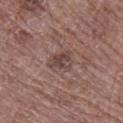Assessment: No biopsy was performed on this lesion — it was imaged during a full skin examination and was not determined to be concerning. Image and clinical context: An algorithmic analysis of the crop reported a lesion area of about 6 mm² and a shape-asymmetry score of about 0.35 (0 = symmetric). The analysis additionally found about 8 CIELAB-L* units darker than the surrounding skin and a normalized border contrast of about 7. It also reported border irregularity of about 3.5 on a 0–10 scale, a within-lesion color-variation index near 5/10, and peripheral color asymmetry of about 1.5. A male subject aged approximately 70. The lesion is located on the right lower leg. Measured at roughly 3.5 mm in maximum diameter. This image is a 15 mm lesion crop taken from a total-body photograph.